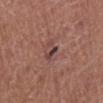No biopsy was performed on this lesion — it was imaged during a full skin examination and was not determined to be concerning. Longest diameter approximately 2.5 mm. From the left lower leg. The tile uses white-light illumination. A 15 mm crop from a total-body photograph taken for skin-cancer surveillance. The subject is a male approximately 75 years of age.